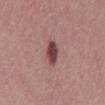follow-up: catalogued during a skin exam; not biopsied | body site: the mid back | lesion size: about 3 mm | patient: male, aged 38–42 | tile lighting: white-light | acquisition: 15 mm crop, total-body photography.